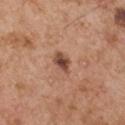follow-up: imaged on a skin check; not biopsied | subject: male, roughly 55 years of age | lighting: white-light | image: total-body-photography crop, ~15 mm field of view | anatomic site: the left upper arm.